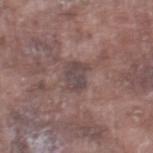workup: catalogued during a skin exam; not biopsied
body site: the right thigh
tile lighting: white-light
image source: ~15 mm crop, total-body skin-cancer survey
TBP lesion metrics: roughly 8 lightness units darker than nearby skin and a lesion-to-skin contrast of about 7.5 (normalized; higher = more distinct); a border-irregularity index near 2.5/10 and a peripheral color-asymmetry measure near 1; a nevus-likeness score of about 0/100
patient: male, aged 73–77
lesion diameter: ~3 mm (longest diameter)